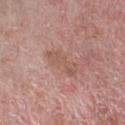The lesion was photographed on a routine skin check and not biopsied; there is no pathology result. This is a white-light tile. Cropped from a total-body skin-imaging series; the visible field is about 15 mm. A female patient about 60 years old. Located on the arm.